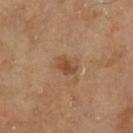<lesion>
<biopsy_status>not biopsied; imaged during a skin examination</biopsy_status>
<image>
  <source>total-body photography crop</source>
  <field_of_view_mm>15</field_of_view_mm>
</image>
<site>left lower leg</site>
<lighting>cross-polarized</lighting>
<automated_metrics>
  <area_mm2_approx>4.0</area_mm2_approx>
  <eccentricity>0.8</eccentricity>
  <shape_asymmetry>0.3</shape_asymmetry>
  <nevus_likeness_0_100>55</nevus_likeness_0_100>
</automated_metrics>
<lesion_size>
  <long_diameter_mm_approx>3.0</long_diameter_mm_approx>
</lesion_size>
<patient>
  <sex>male</sex>
  <age_approx>65</age_approx>
</patient>
</lesion>